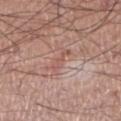<case>
<biopsy_status>not biopsied; imaged during a skin examination</biopsy_status>
<automated_metrics>
  <cielab_L>55</cielab_L>
  <cielab_a>23</cielab_a>
  <cielab_b>26</cielab_b>
  <vs_skin_darker_L>7.0</vs_skin_darker_L>
  <vs_skin_contrast_norm>5.0</vs_skin_contrast_norm>
  <color_variation_0_10>0.0</color_variation_0_10>
  <peripheral_color_asymmetry>0.0</peripheral_color_asymmetry>
</automated_metrics>
<site>leg</site>
<lighting>white-light</lighting>
<lesion_size>
  <long_diameter_mm_approx>3.0</long_diameter_mm_approx>
</lesion_size>
<patient>
  <sex>male</sex>
  <age_approx>45</age_approx>
</patient>
<image>
  <source>total-body photography crop</source>
  <field_of_view_mm>15</field_of_view_mm>
</image>
</case>